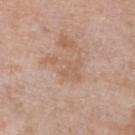Impression:
This lesion was catalogued during total-body skin photography and was not selected for biopsy.
Background:
The lesion is located on the leg. Longest diameter approximately 4.5 mm. A lesion tile, about 15 mm wide, cut from a 3D total-body photograph. A female patient, approximately 70 years of age. The tile uses white-light illumination. Automated tile analysis of the lesion measured an area of roughly 8 mm², an eccentricity of roughly 0.8, and two-axis asymmetry of about 0.65. And it measured a nevus-likeness score of about 0/100 and lesion-presence confidence of about 100/100.